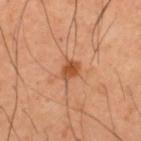  biopsy_status: not biopsied; imaged during a skin examination